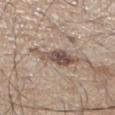Clinical impression:
No biopsy was performed on this lesion — it was imaged during a full skin examination and was not determined to be concerning.
Clinical summary:
The subject is a male in their mid- to late 40s. A 15 mm close-up extracted from a 3D total-body photography capture. The recorded lesion diameter is about 6 mm. Automated image analysis of the tile measured a mean CIELAB color near L≈52 a*≈14 b*≈23 and a lesion-to-skin contrast of about 9 (normalized; higher = more distinct). The lesion is located on the right lower leg.